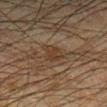Clinical impression: Part of a total-body skin-imaging series; this lesion was reviewed on a skin check and was not flagged for biopsy. Acquisition and patient details: A male patient, aged 33 to 37. The lesion is on the right lower leg. A lesion tile, about 15 mm wide, cut from a 3D total-body photograph. The lesion's longest dimension is about 3 mm.